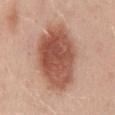Part of a total-body skin-imaging series; this lesion was reviewed on a skin check and was not flagged for biopsy.
The lesion-visualizer software estimated a mean CIELAB color near L≈54 a*≈24 b*≈29 and about 15 CIELAB-L* units darker than the surrounding skin. The analysis additionally found a border-irregularity index near 2/10, internal color variation of about 5.5 on a 0–10 scale, and a peripheral color-asymmetry measure near 1.5.
A 15 mm crop from a total-body photograph taken for skin-cancer surveillance.
The lesion is located on the back.
The subject is a female aged approximately 45.